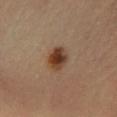follow-up: imaged on a skin check; not biopsied
acquisition: ~15 mm crop, total-body skin-cancer survey
patient: female, in their mid- to late 30s
lesion size: ~3 mm (longest diameter)
site: the left lower leg
tile lighting: cross-polarized illumination
TBP lesion metrics: a lesion color around L≈39 a*≈18 b*≈29 in CIELAB, roughly 13 lightness units darker than nearby skin, and a lesion-to-skin contrast of about 10.5 (normalized; higher = more distinct); a classifier nevus-likeness of about 100/100 and a lesion-detection confidence of about 100/100